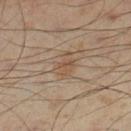Q: Was a biopsy performed?
A: catalogued during a skin exam; not biopsied
Q: Automated lesion metrics?
A: a footprint of about 5.5 mm²; about 7 CIELAB-L* units darker than the surrounding skin and a lesion-to-skin contrast of about 5.5 (normalized; higher = more distinct); a border-irregularity index near 4/10 and a within-lesion color-variation index near 2.5/10; an automated nevus-likeness rating near 40 out of 100 and a lesion-detection confidence of about 100/100
Q: How was this image acquired?
A: 15 mm crop, total-body photography
Q: Where on the body is the lesion?
A: the left lower leg
Q: Lesion size?
A: ~3 mm (longest diameter)
Q: Patient demographics?
A: male, approximately 55 years of age